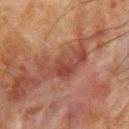Captured during whole-body skin photography for melanoma surveillance; the lesion was not biopsied.